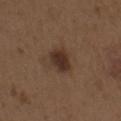Clinical impression:
The lesion was tiled from a total-body skin photograph and was not biopsied.
Clinical summary:
Longest diameter approximately 3 mm. On the arm. This is a white-light tile. A 15 mm close-up extracted from a 3D total-body photography capture. A male subject, aged approximately 70.